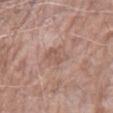<tbp_lesion>
<biopsy_status>not biopsied; imaged during a skin examination</biopsy_status>
<site>right forearm</site>
<image>
  <source>total-body photography crop</source>
  <field_of_view_mm>15</field_of_view_mm>
</image>
<lesion_size>
  <long_diameter_mm_approx>3.5</long_diameter_mm_approx>
</lesion_size>
<patient>
  <sex>male</sex>
  <age_approx>75</age_approx>
</patient>
</tbp_lesion>